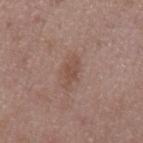Part of a total-body skin-imaging series; this lesion was reviewed on a skin check and was not flagged for biopsy.
A male patient in their 50s.
A 15 mm close-up extracted from a 3D total-body photography capture.
Longest diameter approximately 4 mm.
The tile uses white-light illumination.
An algorithmic analysis of the crop reported a mean CIELAB color near L≈49 a*≈18 b*≈25, a lesion–skin lightness drop of about 7, and a lesion-to-skin contrast of about 6 (normalized; higher = more distinct). The software also gave a border-irregularity index near 4/10, a within-lesion color-variation index near 1.5/10, and peripheral color asymmetry of about 0.5. The software also gave a nevus-likeness score of about 0/100 and lesion-presence confidence of about 100/100.
The lesion is located on the mid back.